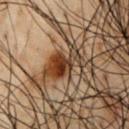workup: no biopsy performed (imaged during a skin exam) | location: the chest | acquisition: total-body-photography crop, ~15 mm field of view | patient: male, about 50 years old.